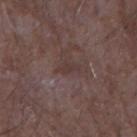Image and clinical context: The patient is a male in their mid- to late 60s. Measured at roughly 2.5 mm in maximum diameter. Imaged with white-light lighting. A region of skin cropped from a whole-body photographic capture, roughly 15 mm wide. On the left forearm.